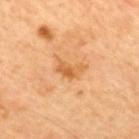Assessment: No biopsy was performed on this lesion — it was imaged during a full skin examination and was not determined to be concerning. Context: A male subject, aged approximately 60. A close-up tile cropped from a whole-body skin photograph, about 15 mm across. Located on the upper back. Captured under cross-polarized illumination. About 3.5 mm across.